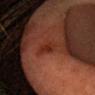patient:
  sex: female
  age_approx: 45
image:
  source: total-body photography crop
  field_of_view_mm: 15
site: head or neck